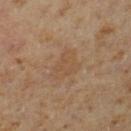{"biopsy_status": "not biopsied; imaged during a skin examination", "image": {"source": "total-body photography crop", "field_of_view_mm": 15}, "patient": {"sex": "male", "age_approx": 60}, "lighting": "cross-polarized", "lesion_size": {"long_diameter_mm_approx": 4.0}, "site": "left lower leg"}